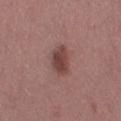Part of a total-body skin-imaging series; this lesion was reviewed on a skin check and was not flagged for biopsy. The patient is a female aged 48–52. This is a white-light tile. About 3.5 mm across. A 15 mm close-up extracted from a 3D total-body photography capture. On the leg.